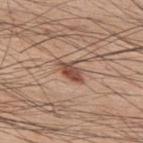This lesion was catalogued during total-body skin photography and was not selected for biopsy.
Imaged with white-light lighting.
The recorded lesion diameter is about 3.5 mm.
An algorithmic analysis of the crop reported a border-irregularity index near 3/10, internal color variation of about 3 on a 0–10 scale, and a peripheral color-asymmetry measure near 1.
On the upper back.
A male subject, about 60 years old.
A 15 mm crop from a total-body photograph taken for skin-cancer surveillance.